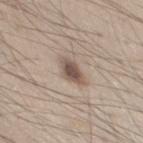{"biopsy_status": "not biopsied; imaged during a skin examination", "lighting": "white-light", "site": "chest", "patient": {"sex": "male", "age_approx": 45}, "lesion_size": {"long_diameter_mm_approx": 3.5}, "image": {"source": "total-body photography crop", "field_of_view_mm": 15}}